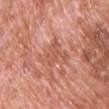The lesion was photographed on a routine skin check and not biopsied; there is no pathology result. The lesion is on the chest. This is a white-light tile. Cropped from a whole-body photographic skin survey; the tile spans about 15 mm. The patient is a male about 70 years old. About 4.5 mm across.